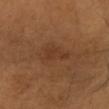  site: head or neck
  patient:
    sex: male
    age_approx: 65
  image:
    source: total-body photography crop
    field_of_view_mm: 15